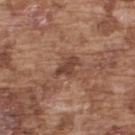Part of a total-body skin-imaging series; this lesion was reviewed on a skin check and was not flagged for biopsy. The lesion's longest dimension is about 3.5 mm. From the upper back. A close-up tile cropped from a whole-body skin photograph, about 15 mm across. The subject is a male in their mid- to late 70s.